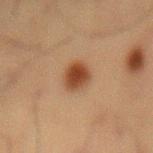{
  "biopsy_status": "not biopsied; imaged during a skin examination",
  "lesion_size": {
    "long_diameter_mm_approx": 3.5
  },
  "site": "mid back",
  "patient": {
    "sex": "male",
    "age_approx": 60
  },
  "automated_metrics": {
    "cielab_L": 35,
    "cielab_a": 19,
    "cielab_b": 28,
    "vs_skin_darker_L": 11.0,
    "vs_skin_contrast_norm": 10.0,
    "border_irregularity_0_10": 1.5,
    "color_variation_0_10": 4.0,
    "peripheral_color_asymmetry": 1.0,
    "nevus_likeness_0_100": 100,
    "lesion_detection_confidence_0_100": 100
  },
  "lighting": "cross-polarized",
  "image": {
    "source": "total-body photography crop",
    "field_of_view_mm": 15
  }
}